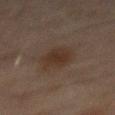Assessment: The lesion was photographed on a routine skin check and not biopsied; there is no pathology result. Context: A close-up tile cropped from a whole-body skin photograph, about 15 mm across. Automated image analysis of the tile measured internal color variation of about 2 on a 0–10 scale and peripheral color asymmetry of about 0.5. The software also gave a classifier nevus-likeness of about 70/100 and a lesion-detection confidence of about 100/100. This is a cross-polarized tile. Located on the mid back. A male subject, aged 43 to 47.